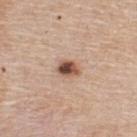  patient:
    sex: male
    age_approx: 55
  lesion_size:
    long_diameter_mm_approx: 2.5
  image:
    source: total-body photography crop
    field_of_view_mm: 15
  site: back
  lighting: white-light